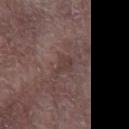Captured during whole-body skin photography for melanoma surveillance; the lesion was not biopsied.
Automated image analysis of the tile measured a lesion area of about 4 mm², an eccentricity of roughly 0.8, and two-axis asymmetry of about 0.3. The analysis additionally found an average lesion color of about L≈38 a*≈16 b*≈18 (CIELAB) and about 6 CIELAB-L* units darker than the surrounding skin. The analysis additionally found a border-irregularity rating of about 3.5/10, a color-variation rating of about 1.5/10, and a peripheral color-asymmetry measure near 0.5.
A roughly 15 mm field-of-view crop from a total-body skin photograph.
This is a white-light tile.
Approximately 3 mm at its widest.
The lesion is located on the left forearm.
A male patient, about 65 years old.